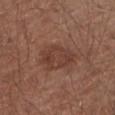No biopsy was performed on this lesion — it was imaged during a full skin examination and was not determined to be concerning.
A 15 mm crop from a total-body photograph taken for skin-cancer surveillance.
The lesion is located on the arm.
A male subject roughly 55 years of age.
Imaged with white-light lighting.